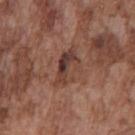  biopsy_status: not biopsied; imaged during a skin examination
  image:
    source: total-body photography crop
    field_of_view_mm: 15
  lesion_size:
    long_diameter_mm_approx: 5.0
  automated_metrics:
    eccentricity: 0.8
    shape_asymmetry: 0.3
    cielab_L: 40
    cielab_a: 20
    cielab_b: 25
    border_irregularity_0_10: 4.0
    color_variation_0_10: 9.5
    peripheral_color_asymmetry: 3.5
  site: chest
  lighting: white-light
  patient:
    sex: male
    age_approx: 75